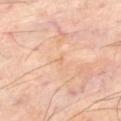| key | value |
|---|---|
| biopsy status | imaged on a skin check; not biopsied |